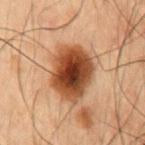The lesion was photographed on a routine skin check and not biopsied; there is no pathology result.
A male subject approximately 50 years of age.
A close-up tile cropped from a whole-body skin photograph, about 15 mm across.
On the abdomen.
Measured at roughly 6 mm in maximum diameter.